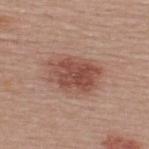biopsy_status: not biopsied; imaged during a skin examination
automated_metrics:
  area_mm2_approx: 16.0
  shape_asymmetry: 0.15
  cielab_L: 49
  cielab_a: 23
  cielab_b: 26
  vs_skin_darker_L: 11.0
  vs_skin_contrast_norm: 8.5
site: upper back
image:
  source: total-body photography crop
  field_of_view_mm: 15
patient:
  sex: male
  age_approx: 40